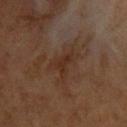workup=no biopsy performed (imaged during a skin exam); patient=male, aged approximately 60; body site=the chest; imaging modality=15 mm crop, total-body photography.